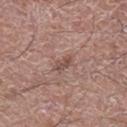Impression: Imaged during a routine full-body skin examination; the lesion was not biopsied and no histopathology is available. Clinical summary: A close-up tile cropped from a whole-body skin photograph, about 15 mm across. Measured at roughly 3 mm in maximum diameter. Automated image analysis of the tile measured a mean CIELAB color near L≈50 a*≈19 b*≈23, about 8 CIELAB-L* units darker than the surrounding skin, and a normalized lesion–skin contrast near 6.5. It also reported border irregularity of about 3 on a 0–10 scale, a color-variation rating of about 2.5/10, and peripheral color asymmetry of about 0.5. The lesion is located on the right lower leg. A male subject about 60 years old.